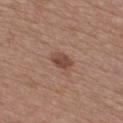No biopsy was performed on this lesion — it was imaged during a full skin examination and was not determined to be concerning.
Imaged with white-light lighting.
A 15 mm close-up tile from a total-body photography series done for melanoma screening.
A female patient aged approximately 40.
The lesion is located on the chest.
About 2.5 mm across.
Automated image analysis of the tile measured a lesion area of about 4.5 mm², a shape eccentricity near 0.7, and a shape-asymmetry score of about 0.15 (0 = symmetric). And it measured a mean CIELAB color near L≈45 a*≈21 b*≈26 and a normalized lesion–skin contrast near 8.